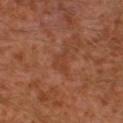Imaged during a routine full-body skin examination; the lesion was not biopsied and no histopathology is available.
Cropped from a whole-body photographic skin survey; the tile spans about 15 mm.
A male patient, approximately 30 years of age.
From the left leg.
Longest diameter approximately 2.5 mm.
Automated image analysis of the tile measured a lesion area of about 2.5 mm² and a shape-asymmetry score of about 0.65 (0 = symmetric). And it measured an average lesion color of about L≈39 a*≈25 b*≈31 (CIELAB), about 5 CIELAB-L* units darker than the surrounding skin, and a normalized lesion–skin contrast near 4.5.